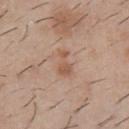Acquisition and patient details:
This image is a 15 mm lesion crop taken from a total-body photograph. The recorded lesion diameter is about 3 mm. Imaged with white-light lighting. The patient is a male about 30 years old. From the chest.